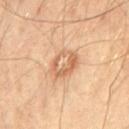• biopsy status: catalogued during a skin exam; not biopsied
• acquisition: ~15 mm crop, total-body skin-cancer survey
• anatomic site: the chest
• TBP lesion metrics: an average lesion color of about L≈64 a*≈21 b*≈36 (CIELAB), a lesion–skin lightness drop of about 10, and a normalized lesion–skin contrast near 6; border irregularity of about 1.5 on a 0–10 scale, internal color variation of about 8.5 on a 0–10 scale, and radial color variation of about 2.5; a nevus-likeness score of about 0/100 and a lesion-detection confidence of about 100/100
• subject: male, about 65 years old
• illumination: cross-polarized